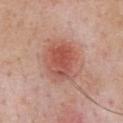Part of a total-body skin-imaging series; this lesion was reviewed on a skin check and was not flagged for biopsy.